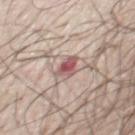Recorded during total-body skin imaging; not selected for excision or biopsy.
Captured under white-light illumination.
A 15 mm close-up tile from a total-body photography series done for melanoma screening.
The lesion's longest dimension is about 3 mm.
A male patient aged 68 to 72.
Located on the chest.